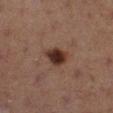Impression: The lesion was tiled from a total-body skin photograph and was not biopsied. Background: Longest diameter approximately 3 mm. A 15 mm close-up tile from a total-body photography series done for melanoma screening. This is a cross-polarized tile. Located on the right lower leg. A female patient approximately 40 years of age. The lesion-visualizer software estimated border irregularity of about 2 on a 0–10 scale and a peripheral color-asymmetry measure near 1. The analysis additionally found an automated nevus-likeness rating near 100 out of 100 and lesion-presence confidence of about 100/100.